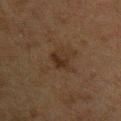Clinical summary:
About 2.5 mm across. From the left upper arm. Captured under cross-polarized illumination. A 15 mm crop from a total-body photograph taken for skin-cancer surveillance. The patient is a male in their 50s.